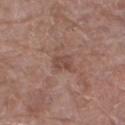Imaged during a routine full-body skin examination; the lesion was not biopsied and no histopathology is available. The lesion's longest dimension is about 2.5 mm. Cropped from a whole-body photographic skin survey; the tile spans about 15 mm. On the right thigh. The patient is a female roughly 70 years of age.